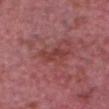notes: total-body-photography surveillance lesion; no biopsy
location: the chest
subject: male, aged 63–67
image source: ~15 mm crop, total-body skin-cancer survey